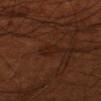Recorded during total-body skin imaging; not selected for excision or biopsy. The total-body-photography lesion software estimated a footprint of about 4 mm², a shape eccentricity near 0.8, and a shape-asymmetry score of about 0.25 (0 = symmetric). It also reported an average lesion color of about L≈19 a*≈18 b*≈24 (CIELAB), roughly 4 lightness units darker than nearby skin, and a lesion-to-skin contrast of about 5.5 (normalized; higher = more distinct). The analysis additionally found internal color variation of about 2 on a 0–10 scale. The analysis additionally found a classifier nevus-likeness of about 5/100 and a lesion-detection confidence of about 100/100. Approximately 3 mm at its widest. The lesion is on the left forearm. A roughly 15 mm field-of-view crop from a total-body skin photograph. A male patient aged approximately 70.